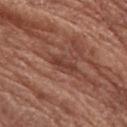biopsy status — no biopsy performed (imaged during a skin exam); image source — total-body-photography crop, ~15 mm field of view; body site — the arm; patient — female, roughly 75 years of age.